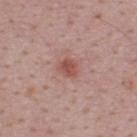Impression:
Part of a total-body skin-imaging series; this lesion was reviewed on a skin check and was not flagged for biopsy.
Image and clinical context:
Captured under white-light illumination. A male subject aged around 50. Automated image analysis of the tile measured about 9 CIELAB-L* units darker than the surrounding skin and a normalized border contrast of about 7. It also reported an automated nevus-likeness rating near 80 out of 100 and a lesion-detection confidence of about 100/100. A 15 mm crop from a total-body photograph taken for skin-cancer surveillance. On the upper back.